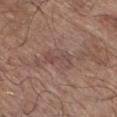A male patient roughly 65 years of age. On the right lower leg. A 15 mm crop from a total-body photograph taken for skin-cancer surveillance.